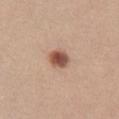biopsy status = catalogued during a skin exam; not biopsied